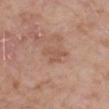{
  "biopsy_status": "not biopsied; imaged during a skin examination",
  "lighting": "white-light",
  "automated_metrics": {
    "cielab_L": 54,
    "cielab_a": 20,
    "cielab_b": 29,
    "vs_skin_contrast_norm": 5.0,
    "border_irregularity_0_10": 5.0,
    "peripheral_color_asymmetry": 0.0
  },
  "image": {
    "source": "total-body photography crop",
    "field_of_view_mm": 15
  },
  "site": "right forearm",
  "patient": {
    "sex": "male",
    "age_approx": 75
  },
  "lesion_size": {
    "long_diameter_mm_approx": 2.5
  }
}